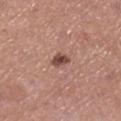Imaged during a routine full-body skin examination; the lesion was not biopsied and no histopathology is available. The lesion-visualizer software estimated roughly 14 lightness units darker than nearby skin and a normalized border contrast of about 9.5. And it measured a color-variation rating of about 3.5/10 and radial color variation of about 1.5. It also reported a lesion-detection confidence of about 100/100. The patient is a female aged 58–62. Imaged with white-light lighting. The lesion's longest dimension is about 2.5 mm. A 15 mm crop from a total-body photograph taken for skin-cancer surveillance. The lesion is located on the right lower leg.